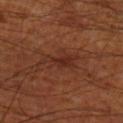Part of a total-body skin-imaging series; this lesion was reviewed on a skin check and was not flagged for biopsy.
The tile uses cross-polarized illumination.
From the leg.
A region of skin cropped from a whole-body photographic capture, roughly 15 mm wide.
The total-body-photography lesion software estimated roughly 6 lightness units darker than nearby skin and a normalized lesion–skin contrast near 6.5. The analysis additionally found a border-irregularity rating of about 6/10.
About 4.5 mm across.
A male patient aged 68 to 72.